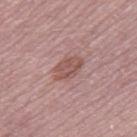Captured during whole-body skin photography for melanoma surveillance; the lesion was not biopsied. Captured under white-light illumination. The patient is a male in their 50s. Cropped from a whole-body photographic skin survey; the tile spans about 15 mm. Approximately 4 mm at its widest. From the right thigh.